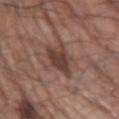<case>
<biopsy_status>not biopsied; imaged during a skin examination</biopsy_status>
<image>
  <source>total-body photography crop</source>
  <field_of_view_mm>15</field_of_view_mm>
</image>
<site>right upper arm</site>
<lesion_size>
  <long_diameter_mm_approx>4.0</long_diameter_mm_approx>
</lesion_size>
<patient>
  <sex>male</sex>
  <age_approx>65</age_approx>
</patient>
<automated_metrics>
  <area_mm2_approx>8.0</area_mm2_approx>
  <eccentricity>0.55</eccentricity>
  <border_irregularity_0_10>4.0</border_irregularity_0_10>
  <color_variation_0_10>4.0</color_variation_0_10>
  <nevus_likeness_0_100>55</nevus_likeness_0_100>
  <lesion_detection_confidence_0_100>100</lesion_detection_confidence_0_100>
</automated_metrics>
<lighting>white-light</lighting>
</case>